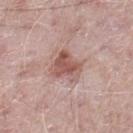Clinical impression: The lesion was photographed on a routine skin check and not biopsied; there is no pathology result. Clinical summary: From the left thigh. A male subject, in their 50s. A region of skin cropped from a whole-body photographic capture, roughly 15 mm wide. The lesion's longest dimension is about 3.5 mm.